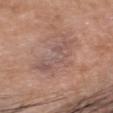Part of a total-body skin-imaging series; this lesion was reviewed on a skin check and was not flagged for biopsy. Cropped from a total-body skin-imaging series; the visible field is about 15 mm. The lesion is located on the head or neck. The recorded lesion diameter is about 5.5 mm. Captured under white-light illumination. A male patient aged 53–57.